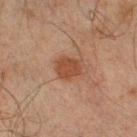This lesion was catalogued during total-body skin photography and was not selected for biopsy. The tile uses cross-polarized illumination. A roughly 15 mm field-of-view crop from a total-body skin photograph. On the right lower leg. The patient is a male approximately 45 years of age.